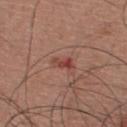– biopsy status — no biopsy performed (imaged during a skin exam)
– body site — the chest
– patient — male, in their mid-30s
– lighting — white-light illumination
– image source — ~15 mm tile from a whole-body skin photo